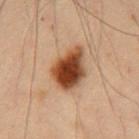Context:
Imaged with cross-polarized lighting. A male subject aged around 55. The lesion is located on the front of the torso. Approximately 5 mm at its widest. A roughly 15 mm field-of-view crop from a total-body skin photograph.